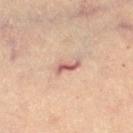Recorded during total-body skin imaging; not selected for excision or biopsy. A female subject aged approximately 45. The lesion is located on the right thigh. Cropped from a whole-body photographic skin survey; the tile spans about 15 mm.